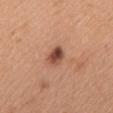follow-up: imaged on a skin check; not biopsied | image source: total-body-photography crop, ~15 mm field of view | patient: female, in their mid-50s | site: the upper back.